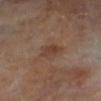{
  "biopsy_status": "not biopsied; imaged during a skin examination",
  "lighting": "cross-polarized",
  "patient": {
    "sex": "male",
    "age_approx": 65
  },
  "site": "right lower leg",
  "image": {
    "source": "total-body photography crop",
    "field_of_view_mm": 15
  },
  "lesion_size": {
    "long_diameter_mm_approx": 2.5
  }
}